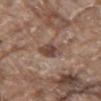Assessment: Imaged during a routine full-body skin examination; the lesion was not biopsied and no histopathology is available. Background: A region of skin cropped from a whole-body photographic capture, roughly 15 mm wide. A male patient aged 78–82. From the mid back.